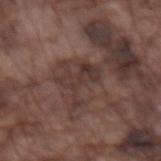| key | value |
|---|---|
| lesion diameter | ≈5 mm |
| TBP lesion metrics | a lesion color around L≈35 a*≈17 b*≈19 in CIELAB, a lesion–skin lightness drop of about 8, and a normalized lesion–skin contrast near 7.5; an automated nevus-likeness rating near 0 out of 100 and lesion-presence confidence of about 55/100 |
| illumination | white-light |
| subject | male, in their mid- to late 70s |
| location | the left forearm |
| acquisition | ~15 mm tile from a whole-body skin photo |